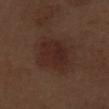Impression:
No biopsy was performed on this lesion — it was imaged during a full skin examination and was not determined to be concerning.
Context:
Imaged with white-light lighting. A male subject, aged around 70. A 15 mm crop from a total-body photograph taken for skin-cancer surveillance. On the left thigh.